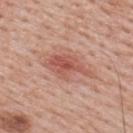Clinical summary:
An algorithmic analysis of the crop reported a lesion area of about 10 mm². It also reported a border-irregularity rating of about 4.5/10, internal color variation of about 4 on a 0–10 scale, and peripheral color asymmetry of about 1. Approximately 5.5 mm at its widest. Located on the upper back. The tile uses white-light illumination. A male subject, approximately 40 years of age. A 15 mm close-up extracted from a 3D total-body photography capture.